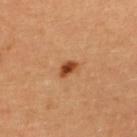• workup — total-body-photography surveillance lesion; no biopsy
• illumination — cross-polarized
• site — the upper back
• patient — female, about 35 years old
• image-analysis metrics — a footprint of about 3 mm²; a classifier nevus-likeness of about 95/100 and a detector confidence of about 100 out of 100 that the crop contains a lesion
• lesion size — ≈2.5 mm
• acquisition — 15 mm crop, total-body photography